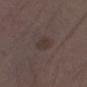Impression: The lesion was photographed on a routine skin check and not biopsied; there is no pathology result. Background: A lesion tile, about 15 mm wide, cut from a 3D total-body photograph. The patient is a female about 35 years old. The lesion-visualizer software estimated a footprint of about 4.5 mm², an outline eccentricity of about 0.75 (0 = round, 1 = elongated), and two-axis asymmetry of about 0.15. It also reported a mean CIELAB color near L≈34 a*≈13 b*≈17 and a lesion–skin lightness drop of about 5. And it measured a nevus-likeness score of about 0/100 and lesion-presence confidence of about 100/100. The lesion's longest dimension is about 3 mm. Captured under white-light illumination. Located on the leg.